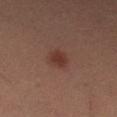Q: Was a biopsy performed?
A: catalogued during a skin exam; not biopsied
Q: What are the patient's age and sex?
A: female, aged 33 to 37
Q: Lesion size?
A: about 2.5 mm
Q: What did automated image analysis measure?
A: a mean CIELAB color near L≈34 a*≈22 b*≈23, roughly 8 lightness units darker than nearby skin, and a normalized border contrast of about 7.5; a border-irregularity rating of about 1.5/10, a color-variation rating of about 1.5/10, and radial color variation of about 0.5; a detector confidence of about 100 out of 100 that the crop contains a lesion
Q: How was the tile lit?
A: cross-polarized illumination
Q: What is the anatomic site?
A: the left lower leg
Q: What kind of image is this?
A: 15 mm crop, total-body photography